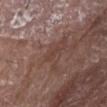notes: total-body-photography surveillance lesion; no biopsy | patient: male, in their 80s | illumination: white-light | diameter: ≈2.5 mm | image-analysis metrics: an area of roughly 2.5 mm², an outline eccentricity of about 0.95 (0 = round, 1 = elongated), and a symmetry-axis asymmetry near 0.4; an average lesion color of about L≈41 a*≈19 b*≈23 (CIELAB) and a normalized lesion–skin contrast near 4.5; a border-irregularity index near 4.5/10 and a within-lesion color-variation index near 0/10; an automated nevus-likeness rating near 0 out of 100 and a detector confidence of about 60 out of 100 that the crop contains a lesion | image source: total-body-photography crop, ~15 mm field of view | site: the head or neck.